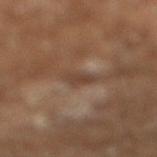follow-up: no biopsy performed (imaged during a skin exam) | illumination: cross-polarized | lesion size: ≈2.5 mm | acquisition: ~15 mm crop, total-body skin-cancer survey | image-analysis metrics: a lesion area of about 3 mm², an eccentricity of roughly 0.8, and a shape-asymmetry score of about 0.4 (0 = symmetric); a border-irregularity index near 4/10, a color-variation rating of about 1.5/10, and radial color variation of about 0.5 | subject: male, approximately 65 years of age.